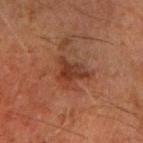Background:
This is a cross-polarized tile. The lesion is located on the right forearm. A male patient aged 58–62. The lesion's longest dimension is about 4 mm. A close-up tile cropped from a whole-body skin photograph, about 15 mm across.